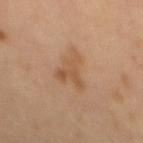The lesion-visualizer software estimated an area of roughly 8.5 mm² and two-axis asymmetry of about 0.6. The software also gave an average lesion color of about L≈51 a*≈19 b*≈33 (CIELAB), roughly 7 lightness units darker than nearby skin, and a lesion-to-skin contrast of about 6 (normalized; higher = more distinct). The software also gave a nevus-likeness score of about 0/100.
The subject is a male aged around 60.
This image is a 15 mm lesion crop taken from a total-body photograph.
The lesion is located on the back.
Longest diameter approximately 5.5 mm.
The tile uses cross-polarized illumination.